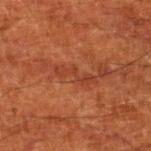biopsy status: no biopsy performed (imaged during a skin exam) | subject: male, in their 80s | site: the right lower leg | acquisition: 15 mm crop, total-body photography.